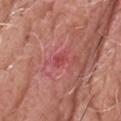Acquisition and patient details: A male subject roughly 60 years of age. Automated image analysis of the tile measured an area of roughly 1.5 mm², a shape eccentricity near 0.8, and a symmetry-axis asymmetry near 0.35. The software also gave a lesion color around L≈47 a*≈38 b*≈25 in CIELAB, roughly 7 lightness units darker than nearby skin, and a normalized border contrast of about 5.5. A close-up tile cropped from a whole-body skin photograph, about 15 mm across. Longest diameter approximately 2 mm. From the head or neck. Imaged with white-light lighting. Diagnosis: The lesion was biopsied, and histopathology showed a superficial basal cell carcinoma, classified as a malignant lesion.